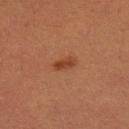Captured during whole-body skin photography for melanoma surveillance; the lesion was not biopsied. The subject is a female aged around 40. A 15 mm close-up extracted from a 3D total-body photography capture. On the right thigh.